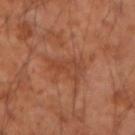Context: Located on the left forearm. Automated tile analysis of the lesion measured a footprint of about 8 mm², a shape eccentricity near 0.75, and a symmetry-axis asymmetry near 0.45. And it measured a border-irregularity rating of about 5.5/10, internal color variation of about 3.5 on a 0–10 scale, and radial color variation of about 1.5. And it measured an automated nevus-likeness rating near 0 out of 100. Captured under cross-polarized illumination. A 15 mm crop from a total-body photograph taken for skin-cancer surveillance. A male patient, aged 53 to 57.